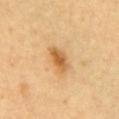Findings:
* follow-up · total-body-photography surveillance lesion; no biopsy
* lesion diameter · ≈3.5 mm
* body site · the chest
* imaging modality · ~15 mm tile from a whole-body skin photo
* tile lighting · cross-polarized illumination
* subject · female, roughly 35 years of age